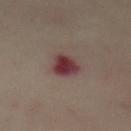Assessment: The lesion was tiled from a total-body skin photograph and was not biopsied. Context: A 15 mm crop from a total-body photograph taken for skin-cancer surveillance. The patient is a female in their mid- to late 50s. From the abdomen. The tile uses cross-polarized illumination. Automated image analysis of the tile measured an automated nevus-likeness rating near 0 out of 100 and a detector confidence of about 100 out of 100 that the crop contains a lesion.